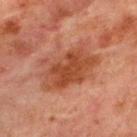  image:
    source: total-body photography crop
    field_of_view_mm: 15
  site: chest
  patient:
    sex: male
    age_approx: 65
  lesion_size:
    long_diameter_mm_approx: 7.0
  automated_metrics:
    area_mm2_approx: 22.0
    eccentricity: 0.8
    shape_asymmetry: 0.3
    cielab_L: 38
    cielab_a: 23
    cielab_b: 29
    vs_skin_darker_L: 9.0
    vs_skin_contrast_norm: 8.0